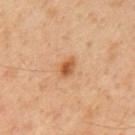{
  "lesion_size": {
    "long_diameter_mm_approx": 2.5
  },
  "image": {
    "source": "total-body photography crop",
    "field_of_view_mm": 15
  },
  "site": "mid back",
  "lighting": "cross-polarized",
  "automated_metrics": {
    "cielab_L": 51,
    "cielab_a": 23,
    "cielab_b": 37,
    "vs_skin_darker_L": 11.0,
    "vs_skin_contrast_norm": 8.5,
    "border_irregularity_0_10": 2.0,
    "color_variation_0_10": 3.5,
    "peripheral_color_asymmetry": 1.0,
    "lesion_detection_confidence_0_100": 100
  },
  "patient": {
    "sex": "male",
    "age_approx": 60
  }
}